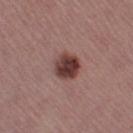biopsy status = imaged on a skin check; not biopsied | tile lighting = white-light | body site = the left thigh | subject = female, about 55 years old | imaging modality = ~15 mm tile from a whole-body skin photo | diameter = about 3 mm | automated lesion analysis = an average lesion color of about L≈39 a*≈22 b*≈20 (CIELAB), about 15 CIELAB-L* units darker than the surrounding skin, and a normalized lesion–skin contrast near 12.